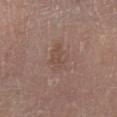This lesion was catalogued during total-body skin photography and was not selected for biopsy.